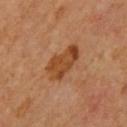notes = no biopsy performed (imaged during a skin exam)
patient = male, aged approximately 65
acquisition = ~15 mm crop, total-body skin-cancer survey
size = ≈5 mm
site = the left upper arm
lighting = cross-polarized illumination